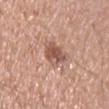biopsy_status: not biopsied; imaged during a skin examination
lesion_size:
  long_diameter_mm_approx: 3.5
automated_metrics:
  area_mm2_approx: 7.0
  shape_asymmetry: 0.25
  color_variation_0_10: 4.0
  peripheral_color_asymmetry: 1.0
  nevus_likeness_0_100: 80
  lesion_detection_confidence_0_100: 100
lighting: white-light
site: left upper arm
patient:
  sex: male
  age_approx: 40
image:
  source: total-body photography crop
  field_of_view_mm: 15